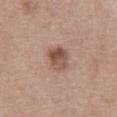workup = catalogued during a skin exam; not biopsied | patient = male, about 60 years old | image = total-body-photography crop, ~15 mm field of view | site = the abdomen | illumination = white-light illumination | lesion size = ~3.5 mm (longest diameter).